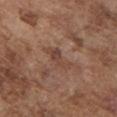<record>
<biopsy_status>not biopsied; imaged during a skin examination</biopsy_status>
<patient>
  <sex>male</sex>
  <age_approx>75</age_approx>
</patient>
<automated_metrics>
  <border_irregularity_0_10>5.5</border_irregularity_0_10>
  <color_variation_0_10>1.5</color_variation_0_10>
  <peripheral_color_asymmetry>0.5</peripheral_color_asymmetry>
  <nevus_likeness_0_100>0</nevus_likeness_0_100>
  <lesion_detection_confidence_0_100>95</lesion_detection_confidence_0_100>
</automated_metrics>
<image>
  <source>total-body photography crop</source>
  <field_of_view_mm>15</field_of_view_mm>
</image>
<site>chest</site>
<lesion_size>
  <long_diameter_mm_approx>4.0</long_diameter_mm_approx>
</lesion_size>
</record>